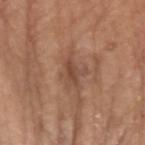Part of a total-body skin-imaging series; this lesion was reviewed on a skin check and was not flagged for biopsy. Located on the left forearm. The patient is a female in their mid-70s. Longest diameter approximately 3 mm. Automated tile analysis of the lesion measured a within-lesion color-variation index near 3.5/10 and a peripheral color-asymmetry measure near 1. Imaged with white-light lighting. A region of skin cropped from a whole-body photographic capture, roughly 15 mm wide.